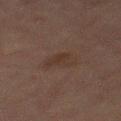Captured during whole-body skin photography for melanoma surveillance; the lesion was not biopsied.